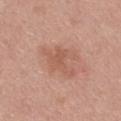follow-up: imaged on a skin check; not biopsied | image: 15 mm crop, total-body photography | body site: the chest | automated lesion analysis: an eccentricity of roughly 0.65 and two-axis asymmetry of about 0.5 | subject: female, about 35 years old | tile lighting: white-light | lesion diameter: ≈4.5 mm.